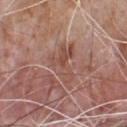biopsy status = catalogued during a skin exam; not biopsied
location = the chest
lighting = white-light illumination
size = ≈5 mm
image source = ~15 mm tile from a whole-body skin photo
patient = male, aged around 65